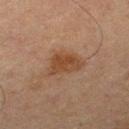Impression:
Imaged during a routine full-body skin examination; the lesion was not biopsied and no histopathology is available.
Acquisition and patient details:
About 4.5 mm across. The total-body-photography lesion software estimated a mean CIELAB color near L≈36 a*≈17 b*≈27, roughly 7 lightness units darker than nearby skin, and a normalized border contrast of about 7.5. It also reported a border-irregularity index near 3.5/10, internal color variation of about 2.5 on a 0–10 scale, and peripheral color asymmetry of about 1. It also reported a lesion-detection confidence of about 100/100. A 15 mm crop from a total-body photograph taken for skin-cancer surveillance. On the right thigh. The patient is a male aged 63–67.